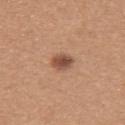Impression: Captured during whole-body skin photography for melanoma surveillance; the lesion was not biopsied. Image and clinical context: The recorded lesion diameter is about 3 mm. The subject is a female about 30 years old. The tile uses white-light illumination. Located on the upper back. A 15 mm crop from a total-body photograph taken for skin-cancer surveillance. Automated tile analysis of the lesion measured a lesion area of about 5 mm², an outline eccentricity of about 0.7 (0 = round, 1 = elongated), and two-axis asymmetry of about 0.15.